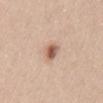Recorded during total-body skin imaging; not selected for excision or biopsy. On the mid back. A region of skin cropped from a whole-body photographic capture, roughly 15 mm wide. An algorithmic analysis of the crop reported a classifier nevus-likeness of about 95/100. The patient is a female in their 50s.